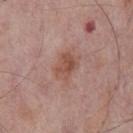Recorded during total-body skin imaging; not selected for excision or biopsy.
The recorded lesion diameter is about 4 mm.
The patient is a male aged 73 to 77.
This is a white-light tile.
A region of skin cropped from a whole-body photographic capture, roughly 15 mm wide.
The total-body-photography lesion software estimated a footprint of about 8 mm² and an outline eccentricity of about 0.7 (0 = round, 1 = elongated). The analysis additionally found a lesion color around L≈51 a*≈22 b*≈27 in CIELAB and a lesion–skin lightness drop of about 8. The analysis additionally found a nevus-likeness score of about 15/100 and lesion-presence confidence of about 100/100.
On the front of the torso.